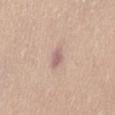Part of a total-body skin-imaging series; this lesion was reviewed on a skin check and was not flagged for biopsy.
A close-up tile cropped from a whole-body skin photograph, about 15 mm across.
Approximately 2.5 mm at its widest.
The total-body-photography lesion software estimated a lesion area of about 3.5 mm², an outline eccentricity of about 0.8 (0 = round, 1 = elongated), and a symmetry-axis asymmetry near 0.2. The analysis additionally found an average lesion color of about L≈62 a*≈19 b*≈22 (CIELAB) and a lesion–skin lightness drop of about 10.
Located on the abdomen.
A female subject about 50 years old.